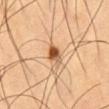notes: imaged on a skin check; not biopsied | imaging modality: ~15 mm crop, total-body skin-cancer survey | size: ≈3 mm | location: the abdomen | patient: male, aged 58–62 | TBP lesion metrics: a mean CIELAB color near L≈55 a*≈23 b*≈38, roughly 16 lightness units darker than nearby skin, and a normalized border contrast of about 10.5; a nevus-likeness score of about 100/100.